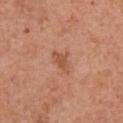| feature | finding |
|---|---|
| workup | imaged on a skin check; not biopsied |
| image-analysis metrics | roughly 8 lightness units darker than nearby skin and a lesion-to-skin contrast of about 6 (normalized; higher = more distinct); a color-variation rating of about 1/10 and peripheral color asymmetry of about 0.5; an automated nevus-likeness rating near 0 out of 100 and a detector confidence of about 100 out of 100 that the crop contains a lesion |
| image source | total-body-photography crop, ~15 mm field of view |
| diameter | about 3 mm |
| subject | male, approximately 70 years of age |
| tile lighting | white-light |
| site | the upper back |